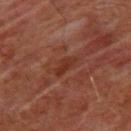Context: About 3 mm across. Automated tile analysis of the lesion measured an area of roughly 3.5 mm², an eccentricity of roughly 0.9, and a symmetry-axis asymmetry near 0.3. Imaged with cross-polarized lighting. A 15 mm crop from a total-body photograph taken for skin-cancer surveillance. The subject is a male aged approximately 60. The lesion is located on the chest.